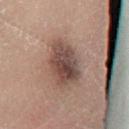Case summary:
• biopsy status · catalogued during a skin exam; not biopsied
• diameter · about 5 mm
• automated lesion analysis · an automated nevus-likeness rating near 70 out of 100 and a detector confidence of about 100 out of 100 that the crop contains a lesion
• anatomic site · the left lower leg
• acquisition · ~15 mm tile from a whole-body skin photo
• subject · male, roughly 70 years of age